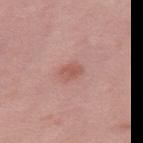biopsy status = no biopsy performed (imaged during a skin exam)
image-analysis metrics = a footprint of about 4 mm², a shape eccentricity near 0.75, and a symmetry-axis asymmetry near 0.35; a lesion color around L≈55 a*≈24 b*≈26 in CIELAB, about 8 CIELAB-L* units darker than the surrounding skin, and a normalized lesion–skin contrast near 6; border irregularity of about 3 on a 0–10 scale and internal color variation of about 2 on a 0–10 scale; an automated nevus-likeness rating near 45 out of 100 and a detector confidence of about 100 out of 100 that the crop contains a lesion
location = the right thigh
patient = female, roughly 40 years of age
diameter = ~2.5 mm (longest diameter)
tile lighting = white-light illumination
image = 15 mm crop, total-body photography